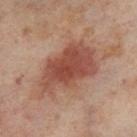Assessment:
Recorded during total-body skin imaging; not selected for excision or biopsy.
Clinical summary:
This is a cross-polarized tile. The subject is a female approximately 55 years of age. Cropped from a whole-body photographic skin survey; the tile spans about 15 mm. Automated image analysis of the tile measured a footprint of about 26 mm², a shape eccentricity near 0.8, and two-axis asymmetry of about 0.3. From the right thigh. The lesion's longest dimension is about 8 mm.